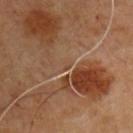Clinical impression: The lesion was photographed on a routine skin check and not biopsied; there is no pathology result. Clinical summary: A male patient approximately 50 years of age. Longest diameter approximately 1 mm. The tile uses cross-polarized illumination. A region of skin cropped from a whole-body photographic capture, roughly 15 mm wide. Automated image analysis of the tile measured an outline eccentricity of about 0.65 (0 = round, 1 = elongated) and two-axis asymmetry of about 0.45. It also reported an average lesion color of about L≈46 a*≈18 b*≈31 (CIELAB), a lesion–skin lightness drop of about 4, and a lesion-to-skin contrast of about 3.5 (normalized; higher = more distinct). And it measured a border-irregularity index near 4/10, a within-lesion color-variation index near 0/10, and peripheral color asymmetry of about 0. The lesion is on the chest.